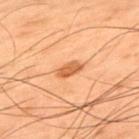Recorded during total-body skin imaging; not selected for excision or biopsy. Automated image analysis of the tile measured a border-irregularity rating of about 1.5/10, a color-variation rating of about 2.5/10, and peripheral color asymmetry of about 1. The patient is a male aged approximately 50. Approximately 3 mm at its widest. Captured under cross-polarized illumination. This image is a 15 mm lesion crop taken from a total-body photograph. The lesion is on the upper back.